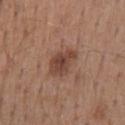Q: Was this lesion biopsied?
A: total-body-photography surveillance lesion; no biopsy
Q: What did automated image analysis measure?
A: a footprint of about 7.5 mm², an eccentricity of roughly 0.8, and a symmetry-axis asymmetry near 0.25; a lesion color around L≈43 a*≈21 b*≈26 in CIELAB and a lesion-to-skin contrast of about 8.5 (normalized; higher = more distinct)
Q: What kind of image is this?
A: total-body-photography crop, ~15 mm field of view
Q: How large is the lesion?
A: ≈4 mm
Q: What are the patient's age and sex?
A: male, aged around 60
Q: How was the tile lit?
A: white-light illumination
Q: Where on the body is the lesion?
A: the mid back